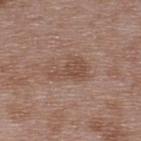The tile uses white-light illumination.
About 4.5 mm across.
The patient is a male aged 48 to 52.
Located on the upper back.
A 15 mm close-up extracted from a 3D total-body photography capture.